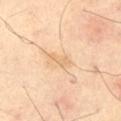Captured during whole-body skin photography for melanoma surveillance; the lesion was not biopsied. Captured under cross-polarized illumination. Cropped from a whole-body photographic skin survey; the tile spans about 15 mm. From the left thigh.